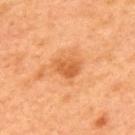Impression:
The lesion was photographed on a routine skin check and not biopsied; there is no pathology result.
Image and clinical context:
A male patient about 50 years old. About 3 mm across. The total-body-photography lesion software estimated an area of roughly 6 mm². The analysis additionally found a mean CIELAB color near L≈60 a*≈30 b*≈46, a lesion–skin lightness drop of about 10, and a lesion-to-skin contrast of about 7 (normalized; higher = more distinct). Captured under cross-polarized illumination. From the upper back. A lesion tile, about 15 mm wide, cut from a 3D total-body photograph.